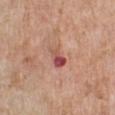No biopsy was performed on this lesion — it was imaged during a full skin examination and was not determined to be concerning. The lesion-visualizer software estimated border irregularity of about 3 on a 0–10 scale and a within-lesion color-variation index near 10/10. A male patient approximately 80 years of age. This is a white-light tile. Located on the right lower leg. Measured at roughly 3 mm in maximum diameter. Cropped from a whole-body photographic skin survey; the tile spans about 15 mm.